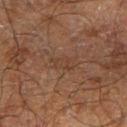{
  "biopsy_status": "not biopsied; imaged during a skin examination",
  "site": "arm",
  "image": {
    "source": "total-body photography crop",
    "field_of_view_mm": 15
  },
  "patient": {
    "sex": "male",
    "age_approx": 70
  }
}